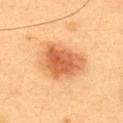Impression:
Recorded during total-body skin imaging; not selected for excision or biopsy.
Acquisition and patient details:
A 15 mm close-up tile from a total-body photography series done for melanoma screening. About 5.5 mm across. From the upper back. Captured under cross-polarized illumination. A female patient aged 28 to 32.